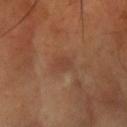This lesion was catalogued during total-body skin photography and was not selected for biopsy.
Automated tile analysis of the lesion measured a nevus-likeness score of about 0/100 and a detector confidence of about 100 out of 100 that the crop contains a lesion.
The tile uses cross-polarized illumination.
About 2.5 mm across.
A region of skin cropped from a whole-body photographic capture, roughly 15 mm wide.
The patient is a male roughly 70 years of age.
The lesion is located on the left forearm.